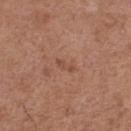• biopsy status · no biopsy performed (imaged during a skin exam)
• imaging modality · 15 mm crop, total-body photography
• diameter · ~2.5 mm (longest diameter)
• anatomic site · the right thigh
• subject · male, about 75 years old